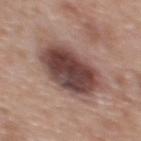{
  "biopsy_status": "not biopsied; imaged during a skin examination",
  "patient": {
    "sex": "female",
    "age_approx": 40
  },
  "image": {
    "source": "total-body photography crop",
    "field_of_view_mm": 15
  },
  "site": "upper back",
  "lighting": "white-light",
  "lesion_size": {
    "long_diameter_mm_approx": 7.0
  },
  "automated_metrics": {
    "color_variation_0_10": 6.5,
    "peripheral_color_asymmetry": 2.0
  }
}